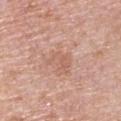| key | value |
|---|---|
| follow-up | no biopsy performed (imaged during a skin exam) |
| subject | male, in their mid-70s |
| acquisition | ~15 mm crop, total-body skin-cancer survey |
| body site | the right upper arm |